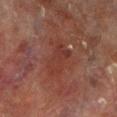<record>
<automated_metrics>
  <area_mm2_approx>10.0</area_mm2_approx>
  <eccentricity>0.85</eccentricity>
  <shape_asymmetry>0.35</shape_asymmetry>
  <cielab_L>29</cielab_L>
  <cielab_a>21</cielab_a>
  <cielab_b>22</cielab_b>
  <vs_skin_darker_L>5.0</vs_skin_darker_L>
  <nevus_likeness_0_100>0</nevus_likeness_0_100>
  <lesion_detection_confidence_0_100>100</lesion_detection_confidence_0_100>
</automated_metrics>
<lighting>cross-polarized</lighting>
<lesion_size>
  <long_diameter_mm_approx>5.0</long_diameter_mm_approx>
</lesion_size>
<patient>
  <sex>male</sex>
  <age_approx>70</age_approx>
</patient>
<site>leg</site>
<image>
  <source>total-body photography crop</source>
  <field_of_view_mm>15</field_of_view_mm>
</image>
</record>